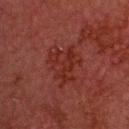Part of a total-body skin-imaging series; this lesion was reviewed on a skin check and was not flagged for biopsy.
The lesion is located on the head or neck.
Cropped from a whole-body photographic skin survey; the tile spans about 15 mm.
A male patient about 60 years old.